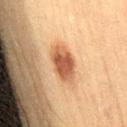No biopsy was performed on this lesion — it was imaged during a full skin examination and was not determined to be concerning.
A male patient, aged around 50.
The tile uses cross-polarized illumination.
A region of skin cropped from a whole-body photographic capture, roughly 15 mm wide.
The lesion is located on the right thigh.